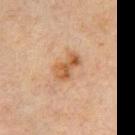Q: Was a biopsy performed?
A: total-body-photography surveillance lesion; no biopsy
Q: Where on the body is the lesion?
A: the chest
Q: What kind of image is this?
A: total-body-photography crop, ~15 mm field of view
Q: How large is the lesion?
A: about 3.5 mm
Q: How was the tile lit?
A: cross-polarized illumination
Q: What are the patient's age and sex?
A: male, about 70 years old